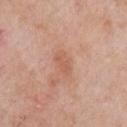<record>
<biopsy_status>not biopsied; imaged during a skin examination</biopsy_status>
</record>